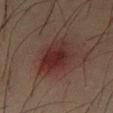| feature | finding |
|---|---|
| biopsy status | total-body-photography surveillance lesion; no biopsy |
| acquisition | 15 mm crop, total-body photography |
| diameter | about 5.5 mm |
| subject | male, aged 33–37 |
| anatomic site | the left thigh |
| tile lighting | cross-polarized |
| automated metrics | a shape-asymmetry score of about 0.2 (0 = symmetric); a border-irregularity rating of about 2/10 and a peripheral color-asymmetry measure near 2; a classifier nevus-likeness of about 85/100 and lesion-presence confidence of about 100/100 |